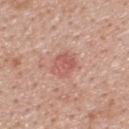follow-up=imaged on a skin check; not biopsied
image=15 mm crop, total-body photography
lesion size=≈3 mm
patient=male, in their 40s
automated metrics=a border-irregularity rating of about 2.5/10, internal color variation of about 2.5 on a 0–10 scale, and peripheral color asymmetry of about 1; a classifier nevus-likeness of about 20/100 and lesion-presence confidence of about 100/100
anatomic site=the upper back